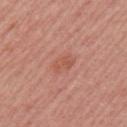follow-up: imaged on a skin check; not biopsied.